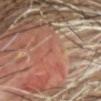Findings:
- follow-up · total-body-photography surveillance lesion; no biopsy
- lesion diameter · ≈1 mm
- image source · ~15 mm crop, total-body skin-cancer survey
- body site · the head or neck
- automated metrics · a footprint of about 0.5 mm², a shape eccentricity near 0.9, and a shape-asymmetry score of about 0.55 (0 = symmetric)
- patient · male, approximately 70 years of age
- lighting · cross-polarized illumination